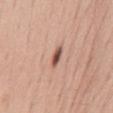Findings:
- biopsy status · total-body-photography surveillance lesion; no biopsy
- location · the mid back
- image · total-body-photography crop, ~15 mm field of view
- patient · female, in their mid- to late 60s